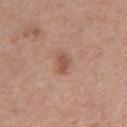Clinical impression: The lesion was tiled from a total-body skin photograph and was not biopsied. Background: A male subject, aged 68–72. A 15 mm close-up extracted from a 3D total-body photography capture. On the abdomen.